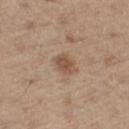Clinical impression:
Recorded during total-body skin imaging; not selected for excision or biopsy.
Context:
Longest diameter approximately 3 mm. A male subject, aged 68 to 72. From the left thigh. A region of skin cropped from a whole-body photographic capture, roughly 15 mm wide.